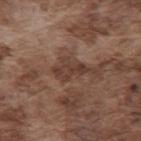Impression: Captured during whole-body skin photography for melanoma surveillance; the lesion was not biopsied. Acquisition and patient details: On the mid back. A male subject approximately 75 years of age. A lesion tile, about 15 mm wide, cut from a 3D total-body photograph.